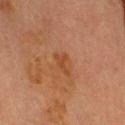biopsy_status: not biopsied; imaged during a skin examination
lighting: cross-polarized
lesion_size:
  long_diameter_mm_approx: 2.5
site: head or neck
image:
  source: total-body photography crop
  field_of_view_mm: 15
patient:
  sex: male
  age_approx: 70